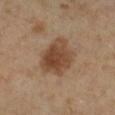Clinical impression: Captured during whole-body skin photography for melanoma surveillance; the lesion was not biopsied. Acquisition and patient details: The lesion-visualizer software estimated roughly 11 lightness units darker than nearby skin and a lesion-to-skin contrast of about 8.5 (normalized; higher = more distinct). It also reported a within-lesion color-variation index near 4.5/10. A lesion tile, about 15 mm wide, cut from a 3D total-body photograph. Located on the leg. Longest diameter approximately 5 mm. Imaged with cross-polarized lighting. A male patient in their mid- to late 60s.